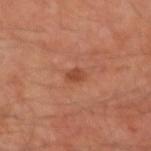workup: total-body-photography surveillance lesion; no biopsy
image-analysis metrics: a nevus-likeness score of about 65/100 and lesion-presence confidence of about 100/100
patient: male, aged 48 to 52
image: ~15 mm crop, total-body skin-cancer survey
size: ≈2 mm
site: the left arm
tile lighting: cross-polarized illumination